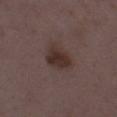Q: What is the lesion's diameter?
A: ~4 mm (longest diameter)
Q: How was the tile lit?
A: white-light illumination
Q: What is the imaging modality?
A: 15 mm crop, total-body photography
Q: Where on the body is the lesion?
A: the left thigh
Q: Patient demographics?
A: female, aged 33 to 37